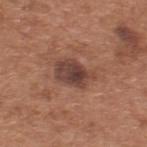<record>
<biopsy_status>not biopsied; imaged during a skin examination</biopsy_status>
<patient>
  <sex>male</sex>
  <age_approx>65</age_approx>
</patient>
<site>upper back</site>
<image>
  <source>total-body photography crop</source>
  <field_of_view_mm>15</field_of_view_mm>
</image>
<lesion_size>
  <long_diameter_mm_approx>4.5</long_diameter_mm_approx>
</lesion_size>
</record>